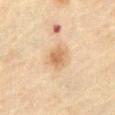biopsy status: imaged on a skin check; not biopsied
subject: male, in their 70s
lighting: cross-polarized
automated lesion analysis: a shape eccentricity near 0.65 and a shape-asymmetry score of about 0.2 (0 = symmetric); a mean CIELAB color near L≈54 a*≈16 b*≈32
image source: total-body-photography crop, ~15 mm field of view
anatomic site: the abdomen
size: about 3 mm